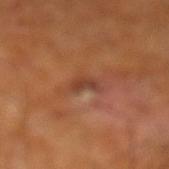Part of a total-body skin-imaging series; this lesion was reviewed on a skin check and was not flagged for biopsy. About 2.5 mm across. A 15 mm close-up extracted from a 3D total-body photography capture. The lesion is located on the left lower leg. A male patient, about 60 years old. This is a cross-polarized tile.